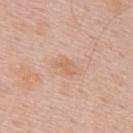The lesion was tiled from a total-body skin photograph and was not biopsied. Cropped from a total-body skin-imaging series; the visible field is about 15 mm. A male subject, aged 53–57. From the upper back.